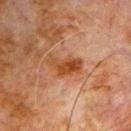notes=imaged on a skin check; not biopsied | anatomic site=the chest | illumination=cross-polarized illumination | image=total-body-photography crop, ~15 mm field of view | subject=male, about 80 years old.